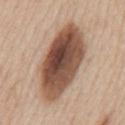Assessment:
The lesion was photographed on a routine skin check and not biopsied; there is no pathology result.
Context:
This is a white-light tile. Cropped from a whole-body photographic skin survey; the tile spans about 15 mm. The lesion is on the mid back. The recorded lesion diameter is about 9.5 mm. A male patient about 60 years old.